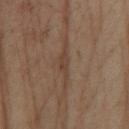The lesion was tiled from a total-body skin photograph and was not biopsied.
The lesion is located on the right lower leg.
The tile uses cross-polarized illumination.
A female patient about 65 years old.
The recorded lesion diameter is about 3 mm.
A region of skin cropped from a whole-body photographic capture, roughly 15 mm wide.